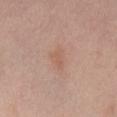The lesion was tiled from a total-body skin photograph and was not biopsied.
Longest diameter approximately 3 mm.
An algorithmic analysis of the crop reported a shape eccentricity near 0.8. The analysis additionally found border irregularity of about 4 on a 0–10 scale, a color-variation rating of about 0.5/10, and peripheral color asymmetry of about 0.5.
A 15 mm close-up tile from a total-body photography series done for melanoma screening.
Imaged with cross-polarized lighting.
A male patient, in their mid- to late 40s.
The lesion is located on the mid back.